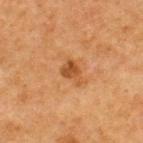Q: Lesion size?
A: about 3 mm
Q: Automated lesion metrics?
A: a lesion area of about 4 mm² and two-axis asymmetry of about 0.4; an average lesion color of about L≈40 a*≈21 b*≈34 (CIELAB); border irregularity of about 4 on a 0–10 scale and radial color variation of about 1; a nevus-likeness score of about 55/100 and a detector confidence of about 100 out of 100 that the crop contains a lesion
Q: Lesion location?
A: the upper back
Q: Patient demographics?
A: male, aged around 75
Q: What kind of image is this?
A: 15 mm crop, total-body photography
Q: Illumination type?
A: cross-polarized